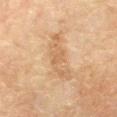Case summary:
– follow-up — total-body-photography surveillance lesion; no biopsy
– patient — female, approximately 80 years of age
– image source — ~15 mm crop, total-body skin-cancer survey
– automated metrics — an area of roughly 9.5 mm² and an eccentricity of roughly 0.9; a classifier nevus-likeness of about 0/100 and a lesion-detection confidence of about 100/100
– site — the right forearm
– lesion diameter — ≈5.5 mm